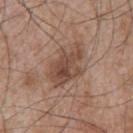biopsy status = catalogued during a skin exam; not biopsied
lesion diameter = ≈6 mm
image = total-body-photography crop, ~15 mm field of view
location = the left upper arm
illumination = white-light illumination
patient = male, aged approximately 65